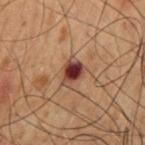biopsy status: imaged on a skin check; not biopsied | acquisition: 15 mm crop, total-body photography | subject: male, aged around 50 | anatomic site: the mid back | automated lesion analysis: an average lesion color of about L≈30 a*≈19 b*≈21 (CIELAB), about 13 CIELAB-L* units darker than the surrounding skin, and a lesion-to-skin contrast of about 13 (normalized; higher = more distinct) | size: ≈3 mm.